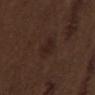biopsy_status: not biopsied; imaged during a skin examination
image:
  source: total-body photography crop
  field_of_view_mm: 15
site: mid back
patient:
  sex: male
  age_approx: 70
lesion_size:
  long_diameter_mm_approx: 3.5
lighting: white-light